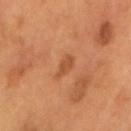Q: Was this lesion biopsied?
A: catalogued during a skin exam; not biopsied
Q: Lesion location?
A: the head or neck
Q: What lighting was used for the tile?
A: cross-polarized illumination
Q: How was this image acquired?
A: ~15 mm tile from a whole-body skin photo
Q: What are the patient's age and sex?
A: male, aged 53–57